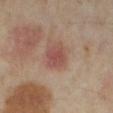Notes:
* workup — imaged on a skin check; not biopsied
* patient — female, in their mid-30s
* imaging modality — ~15 mm tile from a whole-body skin photo
* diameter — about 3 mm
* image-analysis metrics — a border-irregularity index near 1.5/10, internal color variation of about 2.5 on a 0–10 scale, and peripheral color asymmetry of about 0.5; a nevus-likeness score of about 75/100 and lesion-presence confidence of about 100/100
* illumination — cross-polarized
* location — the right lower leg